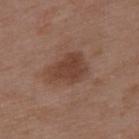Assessment:
No biopsy was performed on this lesion — it was imaged during a full skin examination and was not determined to be concerning.
Background:
Longest diameter approximately 4.5 mm. From the back. Captured under white-light illumination. A close-up tile cropped from a whole-body skin photograph, about 15 mm across. A male patient, approximately 50 years of age.